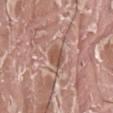Assessment:
Captured during whole-body skin photography for melanoma surveillance; the lesion was not biopsied.
Image and clinical context:
A male subject, approximately 40 years of age. Approximately 3 mm at its widest. The total-body-photography lesion software estimated a lesion area of about 4.5 mm², an outline eccentricity of about 0.75 (0 = round, 1 = elongated), and a symmetry-axis asymmetry near 0.2. The software also gave internal color variation of about 2 on a 0–10 scale. It also reported a detector confidence of about 90 out of 100 that the crop contains a lesion. Cropped from a whole-body photographic skin survey; the tile spans about 15 mm. The lesion is located on the left thigh.